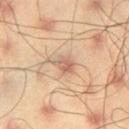follow-up: imaged on a skin check; not biopsied
subject: male, in their mid-40s
location: the left thigh
imaging modality: total-body-photography crop, ~15 mm field of view